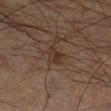notes: total-body-photography surveillance lesion; no biopsy | illumination: cross-polarized | imaging modality: total-body-photography crop, ~15 mm field of view | subject: male, in their mid- to late 60s | anatomic site: the left lower leg | image-analysis metrics: a mean CIELAB color near L≈27 a*≈14 b*≈21 and a lesion-to-skin contrast of about 6.5 (normalized; higher = more distinct).